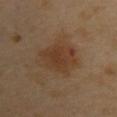No biopsy was performed on this lesion — it was imaged during a full skin examination and was not determined to be concerning.
A 15 mm crop from a total-body photograph taken for skin-cancer surveillance.
Located on the upper back.
A female patient, in their 50s.